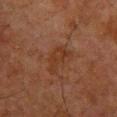Recorded during total-body skin imaging; not selected for excision or biopsy. A male patient aged around 65. The lesion is located on the chest. The recorded lesion diameter is about 4 mm. A 15 mm close-up tile from a total-body photography series done for melanoma screening. Imaged with cross-polarized lighting.